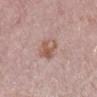Context:
Approximately 4 mm at its widest. Captured under white-light illumination. A close-up tile cropped from a whole-body skin photograph, about 15 mm across. The lesion is located on the back. A male patient, aged around 75. Automated tile analysis of the lesion measured a lesion area of about 7 mm², an outline eccentricity of about 0.85 (0 = round, 1 = elongated), and two-axis asymmetry of about 0.25. The analysis additionally found an average lesion color of about L≈56 a*≈20 b*≈25 (CIELAB), a lesion–skin lightness drop of about 9, and a lesion-to-skin contrast of about 7 (normalized; higher = more distinct). And it measured a border-irregularity rating of about 3/10, a color-variation rating of about 7/10, and radial color variation of about 2.5. It also reported a nevus-likeness score of about 40/100.